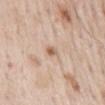Part of a total-body skin-imaging series; this lesion was reviewed on a skin check and was not flagged for biopsy.
The patient is a male aged approximately 65.
A 15 mm close-up tile from a total-body photography series done for melanoma screening.
The lesion is on the back.